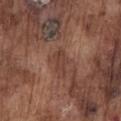• follow-up: no biopsy performed (imaged during a skin exam)
• imaging modality: ~15 mm crop, total-body skin-cancer survey
• site: the front of the torso
• lesion size: ≈3 mm
• patient: male, approximately 75 years of age